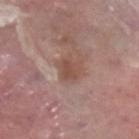Image and clinical context: A male patient, aged around 40. Located on the right lower leg. A 15 mm close-up tile from a total-body photography series done for melanoma screening.